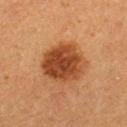The lesion was tiled from a total-body skin photograph and was not biopsied. A female subject, approximately 40 years of age. The total-body-photography lesion software estimated an average lesion color of about L≈36 a*≈22 b*≈32 (CIELAB) and about 12 CIELAB-L* units darker than the surrounding skin. It also reported a border-irregularity index near 1.5/10, internal color variation of about 4.5 on a 0–10 scale, and radial color variation of about 1.5. About 5.5 mm across. The lesion is on the right lower leg. Captured under cross-polarized illumination. A region of skin cropped from a whole-body photographic capture, roughly 15 mm wide.